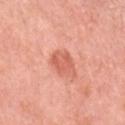Part of a total-body skin-imaging series; this lesion was reviewed on a skin check and was not flagged for biopsy. A female patient, approximately 55 years of age. This is a white-light tile. About 2.5 mm across. A roughly 15 mm field-of-view crop from a total-body skin photograph. The lesion is located on the left upper arm.